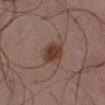Clinical impression:
Captured during whole-body skin photography for melanoma surveillance; the lesion was not biopsied.
Background:
A lesion tile, about 15 mm wide, cut from a 3D total-body photograph. A male patient, roughly 40 years of age. The lesion-visualizer software estimated an eccentricity of roughly 0.6 and a shape-asymmetry score of about 0.15 (0 = symmetric). It also reported a lesion color around L≈39 a*≈19 b*≈24 in CIELAB and a lesion-to-skin contrast of about 9.5 (normalized; higher = more distinct). The lesion is located on the chest. This is a white-light tile. Measured at roughly 3.5 mm in maximum diameter.